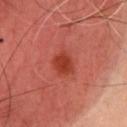workup: imaged on a skin check; not biopsied
patient: male, in their mid-50s
imaging modality: 15 mm crop, total-body photography
tile lighting: cross-polarized
diameter: ~2.5 mm (longest diameter)
anatomic site: the chest
automated metrics: an average lesion color of about L≈40 a*≈36 b*≈34 (CIELAB), about 10 CIELAB-L* units darker than the surrounding skin, and a normalized border contrast of about 8.5; a border-irregularity index near 1.5/10 and radial color variation of about 1; an automated nevus-likeness rating near 95 out of 100 and a lesion-detection confidence of about 100/100